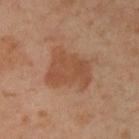Impression:
The lesion was photographed on a routine skin check and not biopsied; there is no pathology result.
Acquisition and patient details:
Measured at roughly 5 mm in maximum diameter. Cropped from a total-body skin-imaging series; the visible field is about 15 mm. Automated tile analysis of the lesion measured a lesion color around L≈50 a*≈22 b*≈33 in CIELAB and a lesion–skin lightness drop of about 8. The analysis additionally found an automated nevus-likeness rating near 55 out of 100 and a detector confidence of about 100 out of 100 that the crop contains a lesion. Captured under cross-polarized illumination. The lesion is on the left lower leg. The subject is a female aged approximately 50.